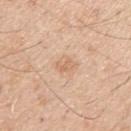The lesion was tiled from a total-body skin photograph and was not biopsied. A lesion tile, about 15 mm wide, cut from a 3D total-body photograph. On the arm. The patient is a male in their mid-30s.